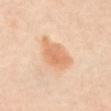Captured during whole-body skin photography for melanoma surveillance; the lesion was not biopsied. This image is a 15 mm lesion crop taken from a total-body photograph. From the abdomen. A female subject, aged 58–62.